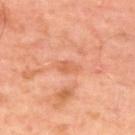The lesion was tiled from a total-body skin photograph and was not biopsied. Located on the upper back. A male subject aged 48–52. Cropped from a whole-body photographic skin survey; the tile spans about 15 mm. The tile uses cross-polarized illumination. Automated tile analysis of the lesion measured about 7 CIELAB-L* units darker than the surrounding skin and a normalized border contrast of about 5.5. And it measured a border-irregularity rating of about 2.5/10 and radial color variation of about 0.5. Approximately 3 mm at its widest.